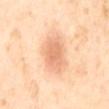Q: What are the patient's age and sex?
A: female, aged 53–57
Q: How was the tile lit?
A: cross-polarized illumination
Q: What did automated image analysis measure?
A: a lesion color around L≈74 a*≈25 b*≈38 in CIELAB, a lesion–skin lightness drop of about 11, and a normalized border contrast of about 6; a border-irregularity rating of about 1.5/10 and peripheral color asymmetry of about 0.5
Q: What is the lesion's diameter?
A: ≈4 mm
Q: What is the anatomic site?
A: the mid back
Q: How was this image acquired?
A: ~15 mm tile from a whole-body skin photo